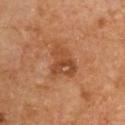biopsy_status: not biopsied; imaged during a skin examination
site: right upper arm
image:
  source: total-body photography crop
  field_of_view_mm: 15
lesion_size:
  long_diameter_mm_approx: 4.5
patient:
  sex: male
  age_approx: 55
lighting: cross-polarized